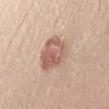<tbp_lesion>
  <biopsy_status>not biopsied; imaged during a skin examination</biopsy_status>
  <image>
    <source>total-body photography crop</source>
    <field_of_view_mm>15</field_of_view_mm>
  </image>
  <lighting>white-light</lighting>
  <lesion_size>
    <long_diameter_mm_approx>4.5</long_diameter_mm_approx>
  </lesion_size>
  <site>left forearm</site>
  <patient>
    <sex>female</sex>
    <age_approx>25</age_approx>
  </patient>
  <automated_metrics>
    <area_mm2_approx>14.0</area_mm2_approx>
    <shape_asymmetry>0.2</shape_asymmetry>
    <vs_skin_darker_L>11.0</vs_skin_darker_L>
    <vs_skin_contrast_norm>7.5</vs_skin_contrast_norm>
    <nevus_likeness_0_100>30</nevus_likeness_0_100>
    <lesion_detection_confidence_0_100>100</lesion_detection_confidence_0_100>
  </automated_metrics>
</tbp_lesion>